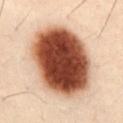Part of a total-body skin-imaging series; this lesion was reviewed on a skin check and was not flagged for biopsy.
A male subject aged 48 to 52.
The recorded lesion diameter is about 9 mm.
A 15 mm crop from a total-body photograph taken for skin-cancer surveillance.
The lesion is located on the abdomen.
Imaged with cross-polarized lighting.
The total-body-photography lesion software estimated an eccentricity of roughly 0.65 and two-axis asymmetry of about 0.1. The software also gave an automated nevus-likeness rating near 100 out of 100.